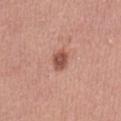Q: Was this lesion biopsied?
A: no biopsy performed (imaged during a skin exam)
Q: Illumination type?
A: white-light illumination
Q: What are the patient's age and sex?
A: female, approximately 50 years of age
Q: What is the anatomic site?
A: the abdomen
Q: Automated lesion metrics?
A: a lesion color around L≈52 a*≈25 b*≈28 in CIELAB; a nevus-likeness score of about 95/100 and a detector confidence of about 100 out of 100 that the crop contains a lesion
Q: What is the lesion's diameter?
A: ≈2.5 mm
Q: What is the imaging modality?
A: total-body-photography crop, ~15 mm field of view